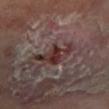The lesion was tiled from a total-body skin photograph and was not biopsied.
Cropped from a total-body skin-imaging series; the visible field is about 15 mm.
Captured under cross-polarized illumination.
The lesion is located on the left lower leg.
A male subject aged around 70.
The recorded lesion diameter is about 5 mm.